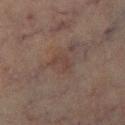Impression:
Imaged during a routine full-body skin examination; the lesion was not biopsied and no histopathology is available.
Context:
The lesion's longest dimension is about 2.5 mm. A female subject aged around 80. Cropped from a total-body skin-imaging series; the visible field is about 15 mm. The lesion-visualizer software estimated an eccentricity of roughly 0.8 and a symmetry-axis asymmetry near 0.45. It also reported a lesion–skin lightness drop of about 4 and a normalized border contrast of about 4.5. And it measured radial color variation of about 0. The lesion is on the right lower leg.